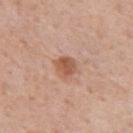notes: catalogued during a skin exam; not biopsied | patient: male, roughly 55 years of age | site: the chest | automated lesion analysis: a footprint of about 5 mm², a shape eccentricity near 0.4, and a symmetry-axis asymmetry near 0.2; border irregularity of about 1.5 on a 0–10 scale and a color-variation rating of about 3.5/10 | acquisition: ~15 mm tile from a whole-body skin photo.